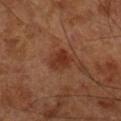Findings:
* notes: total-body-photography surveillance lesion; no biopsy
* acquisition: ~15 mm crop, total-body skin-cancer survey
* subject: roughly 65 years of age
* anatomic site: the leg